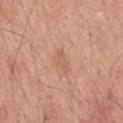This lesion was catalogued during total-body skin photography and was not selected for biopsy. The lesion's longest dimension is about 3 mm. This image is a 15 mm lesion crop taken from a total-body photograph. An algorithmic analysis of the crop reported a mean CIELAB color near L≈60 a*≈22 b*≈33 and a lesion–skin lightness drop of about 6. And it measured border irregularity of about 4 on a 0–10 scale and a within-lesion color-variation index near 1.5/10. Imaged with white-light lighting. The lesion is located on the back. The patient is a male approximately 60 years of age.